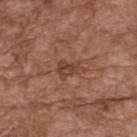Notes:
• biopsy status — no biopsy performed (imaged during a skin exam)
• patient — male, roughly 75 years of age
• automated lesion analysis — a border-irregularity rating of about 5/10 and internal color variation of about 3.5 on a 0–10 scale
• image — 15 mm crop, total-body photography
• diameter — ≈3 mm
• lighting — white-light illumination
• location — the back